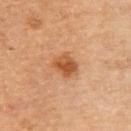The lesion was tiled from a total-body skin photograph and was not biopsied.
Located on the upper back.
A 15 mm close-up tile from a total-body photography series done for melanoma screening.
The subject is a female aged around 60.